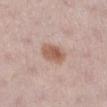notes: total-body-photography surveillance lesion; no biopsy
acquisition: 15 mm crop, total-body photography
subject: female, aged around 30
TBP lesion metrics: a lesion area of about 7.5 mm², an outline eccentricity of about 0.8 (0 = round, 1 = elongated), and a shape-asymmetry score of about 0.2 (0 = symmetric); a lesion color around L≈58 a*≈20 b*≈27 in CIELAB, a lesion–skin lightness drop of about 11, and a normalized lesion–skin contrast near 8
anatomic site: the right lower leg
lesion size: about 4 mm
lighting: white-light illumination